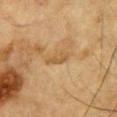Impression: The lesion was tiled from a total-body skin photograph and was not biopsied. Context: From the chest. The patient is a male roughly 85 years of age or older. Automated image analysis of the tile measured an average lesion color of about L≈48 a*≈15 b*≈36 (CIELAB), roughly 7 lightness units darker than nearby skin, and a normalized border contrast of about 6. It also reported peripheral color asymmetry of about 0.5. Imaged with cross-polarized lighting. A roughly 15 mm field-of-view crop from a total-body skin photograph. Measured at roughly 3 mm in maximum diameter.